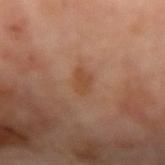{"biopsy_status": "not biopsied; imaged during a skin examination", "site": "left forearm", "lesion_size": {"long_diameter_mm_approx": 2.5}, "lighting": "cross-polarized", "image": {"source": "total-body photography crop", "field_of_view_mm": 15}, "patient": {"sex": "male", "age_approx": 70}}